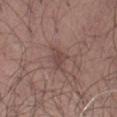Part of a total-body skin-imaging series; this lesion was reviewed on a skin check and was not flagged for biopsy. A male patient aged approximately 50. Imaged with white-light lighting. A roughly 15 mm field-of-view crop from a total-body skin photograph. An algorithmic analysis of the crop reported a border-irregularity index near 3/10, a within-lesion color-variation index near 1.5/10, and peripheral color asymmetry of about 0.5. And it measured a nevus-likeness score of about 0/100 and a lesion-detection confidence of about 95/100. The lesion is located on the left thigh. Longest diameter approximately 3 mm.